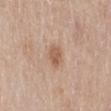  image:
    source: total-body photography crop
    field_of_view_mm: 15
  lesion_size:
    long_diameter_mm_approx: 3.0
  lighting: white-light
  patient:
    sex: female
    age_approx: 60
  site: back